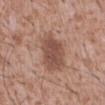biopsy status=total-body-photography surveillance lesion; no biopsy
lesion diameter=about 5.5 mm
image source=total-body-photography crop, ~15 mm field of view
location=the abdomen
patient=male, aged 43–47